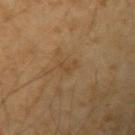The lesion is on the left upper arm. The patient is a male aged approximately 50. This image is a 15 mm lesion crop taken from a total-body photograph.